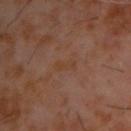Imaged during a routine full-body skin examination; the lesion was not biopsied and no histopathology is available. A male subject, approximately 60 years of age. From the back. Approximately 3 mm at its widest. Cropped from a total-body skin-imaging series; the visible field is about 15 mm.